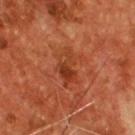  biopsy_status: not biopsied; imaged during a skin examination
  patient:
    sex: male
    age_approx: 55
  automated_metrics:
    area_mm2_approx: 8.0
    eccentricity: 0.85
    shape_asymmetry: 0.35
    cielab_L: 37
    cielab_a: 28
    cielab_b: 35
    vs_skin_darker_L: 7.0
    vs_skin_contrast_norm: 6.5
    nevus_likeness_0_100: 0
    lesion_detection_confidence_0_100: 100
  lighting: cross-polarized
  site: chest
  lesion_size:
    long_diameter_mm_approx: 4.5
  image:
    source: total-body photography crop
    field_of_view_mm: 15